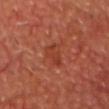follow-up — imaged on a skin check; not biopsied | image source — ~15 mm tile from a whole-body skin photo | lesion diameter — ~3.5 mm (longest diameter) | subject — aged approximately 65 | automated lesion analysis — a normalized border contrast of about 5.5 | location — the head or neck.